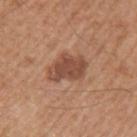{
  "image": {
    "source": "total-body photography crop",
    "field_of_view_mm": 15
  },
  "site": "left upper arm",
  "lighting": "white-light",
  "automated_metrics": {
    "cielab_L": 48,
    "cielab_a": 22,
    "cielab_b": 30,
    "vs_skin_darker_L": 11.0,
    "vs_skin_contrast_norm": 8.5,
    "border_irregularity_0_10": 2.5,
    "color_variation_0_10": 2.5,
    "peripheral_color_asymmetry": 1.0
  },
  "lesion_size": {
    "long_diameter_mm_approx": 4.5
  },
  "patient": {
    "sex": "male",
    "age_approx": 65
  }
}